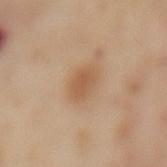Notes:
• workup — imaged on a skin check; not biopsied
• anatomic site — the mid back
• subject — female, aged 53 to 57
• image — 15 mm crop, total-body photography